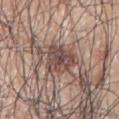On the left arm.
Cropped from a whole-body photographic skin survey; the tile spans about 15 mm.
The lesion's longest dimension is about 4.5 mm.
Automated tile analysis of the lesion measured a detector confidence of about 95 out of 100 that the crop contains a lesion.
The subject is a male aged approximately 70.